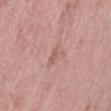workup: no biopsy performed (imaged during a skin exam)
lesion diameter: ~2.5 mm (longest diameter)
imaging modality: ~15 mm tile from a whole-body skin photo
illumination: white-light illumination
patient: male, roughly 70 years of age
anatomic site: the left thigh